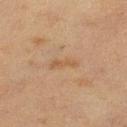workup: imaged on a skin check; not biopsied | lesion size: about 3.5 mm | image: total-body-photography crop, ~15 mm field of view | anatomic site: the left lower leg | lighting: cross-polarized | subject: female, aged approximately 55 | automated metrics: a lesion area of about 3.5 mm², an outline eccentricity of about 0.95 (0 = round, 1 = elongated), and two-axis asymmetry of about 0.45; a lesion color around L≈45 a*≈15 b*≈30 in CIELAB, a lesion–skin lightness drop of about 5, and a normalized border contrast of about 5; a border-irregularity index near 5/10, internal color variation of about 0 on a 0–10 scale, and peripheral color asymmetry of about 0.